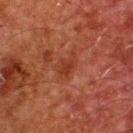<lesion>
<biopsy_status>not biopsied; imaged during a skin examination</biopsy_status>
<patient>
  <sex>male</sex>
  <age_approx>60</age_approx>
</patient>
<lesion_size>
  <long_diameter_mm_approx>3.5</long_diameter_mm_approx>
</lesion_size>
<site>back</site>
<lighting>cross-polarized</lighting>
<image>
  <source>total-body photography crop</source>
  <field_of_view_mm>15</field_of_view_mm>
</image>
<automated_metrics>
  <area_mm2_approx>5.0</area_mm2_approx>
  <eccentricity>0.75</eccentricity>
  <shape_asymmetry>0.35</shape_asymmetry>
  <border_irregularity_0_10>3.5</border_irregularity_0_10>
  <peripheral_color_asymmetry>0.5</peripheral_color_asymmetry>
  <nevus_likeness_0_100>0</nevus_likeness_0_100>
  <lesion_detection_confidence_0_100>100</lesion_detection_confidence_0_100>
</automated_metrics>
</lesion>